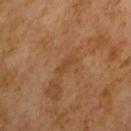– subject — male, aged approximately 65
– tile lighting — cross-polarized
– size — ≈2.5 mm
– image source — ~15 mm tile from a whole-body skin photo